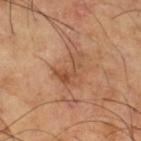Assessment: Imaged during a routine full-body skin examination; the lesion was not biopsied and no histopathology is available. Clinical summary: A male patient, in their mid-60s. A 15 mm crop from a total-body photograph taken for skin-cancer surveillance. The total-body-photography lesion software estimated a border-irregularity index near 7.5/10, a within-lesion color-variation index near 3/10, and peripheral color asymmetry of about 1. The software also gave a detector confidence of about 100 out of 100 that the crop contains a lesion. From the right thigh. Longest diameter approximately 4 mm. The tile uses cross-polarized illumination.